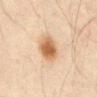| feature | finding |
|---|---|
| body site | the lower back |
| automated lesion analysis | an outline eccentricity of about 0.8 (0 = round, 1 = elongated) and two-axis asymmetry of about 0.15; a mean CIELAB color near L≈52 a*≈17 b*≈32 and a lesion–skin lightness drop of about 12; a border-irregularity index near 1.5/10, internal color variation of about 5 on a 0–10 scale, and peripheral color asymmetry of about 1; a nevus-likeness score of about 100/100 |
| tile lighting | cross-polarized |
| imaging modality | total-body-photography crop, ~15 mm field of view |
| patient | male, aged 68–72 |